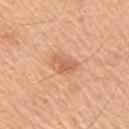<lesion>
<site>right upper arm</site>
<image>
  <source>total-body photography crop</source>
  <field_of_view_mm>15</field_of_view_mm>
</image>
<lighting>white-light</lighting>
<lesion_size>
  <long_diameter_mm_approx>3.0</long_diameter_mm_approx>
</lesion_size>
<patient>
  <sex>male</sex>
  <age_approx>50</age_approx>
</patient>
</lesion>